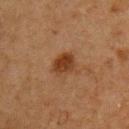notes: no biopsy performed (imaged during a skin exam)
anatomic site: the chest
automated metrics: a within-lesion color-variation index near 3/10 and a peripheral color-asymmetry measure near 1
subject: male, aged approximately 75
image source: ~15 mm crop, total-body skin-cancer survey